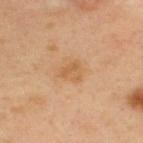Imaged during a routine full-body skin examination; the lesion was not biopsied and no histopathology is available. The subject is a male in their mid- to late 30s. Cropped from a whole-body photographic skin survey; the tile spans about 15 mm. On the upper back. An algorithmic analysis of the crop reported an eccentricity of roughly 0.55. And it measured a lesion color around L≈57 a*≈20 b*≈38 in CIELAB, a lesion–skin lightness drop of about 7, and a lesion-to-skin contrast of about 5.5 (normalized; higher = more distinct). Captured under cross-polarized illumination. The lesion's longest dimension is about 2.5 mm.